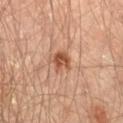Clinical impression:
The lesion was tiled from a total-body skin photograph and was not biopsied.
Context:
Longest diameter approximately 2.5 mm. A region of skin cropped from a whole-body photographic capture, roughly 15 mm wide. The lesion is on the left thigh. A male subject, aged approximately 65.